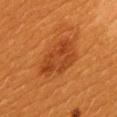Clinical impression: This lesion was catalogued during total-body skin photography and was not selected for biopsy.